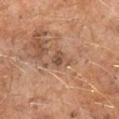biopsy_status: not biopsied; imaged during a skin examination
site: right lower leg
lighting: cross-polarized
image:
  source: total-body photography crop
  field_of_view_mm: 15
patient:
  sex: male
  age_approx: 60
lesion_size:
  long_diameter_mm_approx: 2.5